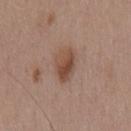{
  "biopsy_status": "not biopsied; imaged during a skin examination",
  "lesion_size": {
    "long_diameter_mm_approx": 4.0
  },
  "image": {
    "source": "total-body photography crop",
    "field_of_view_mm": 15
  },
  "lighting": "white-light",
  "patient": {
    "sex": "male",
    "age_approx": 55
  },
  "site": "chest"
}